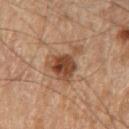No biopsy was performed on this lesion — it was imaged during a full skin examination and was not determined to be concerning. The lesion's longest dimension is about 6 mm. The lesion is on the left upper arm. A 15 mm crop from a total-body photograph taken for skin-cancer surveillance. The patient is a male aged 68 to 72. This is a cross-polarized tile. The lesion-visualizer software estimated an eccentricity of roughly 0.85. And it measured an average lesion color of about L≈34 a*≈16 b*≈25 (CIELAB), about 10 CIELAB-L* units darker than the surrounding skin, and a normalized lesion–skin contrast near 9.